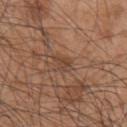The lesion was tiled from a total-body skin photograph and was not biopsied.
About 2.5 mm across.
A lesion tile, about 15 mm wide, cut from a 3D total-body photograph.
The lesion is located on the upper back.
A male patient aged around 45.
Imaged with white-light lighting.